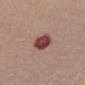Clinical summary:
The lesion-visualizer software estimated a lesion area of about 7 mm², a shape eccentricity near 0.75, and a symmetry-axis asymmetry near 0.15. It also reported a border-irregularity rating of about 1.5/10, internal color variation of about 3.5 on a 0–10 scale, and radial color variation of about 1.5. Captured under white-light illumination. Cropped from a whole-body photographic skin survey; the tile spans about 15 mm. Approximately 3.5 mm at its widest. On the mid back. The patient is a female aged 68 to 72.